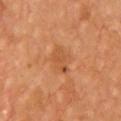Imaged during a routine full-body skin examination; the lesion was not biopsied and no histopathology is available. A 15 mm close-up tile from a total-body photography series done for melanoma screening. The lesion is located on the chest.